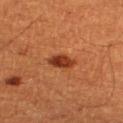Findings:
- notes — imaged on a skin check; not biopsied
- patient — male, approximately 60 years of age
- lesion diameter — about 3 mm
- image source — total-body-photography crop, ~15 mm field of view
- TBP lesion metrics — a lesion–skin lightness drop of about 12; a border-irregularity index near 1.5/10 and peripheral color asymmetry of about 1.5
- body site — the left thigh